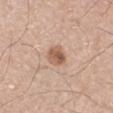Q: Was a biopsy performed?
A: catalogued during a skin exam; not biopsied
Q: What are the patient's age and sex?
A: male, in their mid-60s
Q: How large is the lesion?
A: ~2.5 mm (longest diameter)
Q: What is the anatomic site?
A: the arm
Q: What kind of image is this?
A: ~15 mm crop, total-body skin-cancer survey